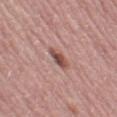biopsy status — no biopsy performed (imaged during a skin exam) | body site — the left thigh | tile lighting — white-light illumination | image — 15 mm crop, total-body photography | image-analysis metrics — a footprint of about 4 mm², an eccentricity of roughly 0.85, and a shape-asymmetry score of about 0.2 (0 = symmetric); a border-irregularity index near 2.5/10, a within-lesion color-variation index near 3/10, and radial color variation of about 1; a classifier nevus-likeness of about 70/100 and lesion-presence confidence of about 100/100 | patient — female, aged 48–52 | lesion diameter — about 3 mm.